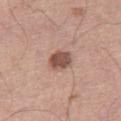Impression: The lesion was photographed on a routine skin check and not biopsied; there is no pathology result. Acquisition and patient details: From the right thigh. The patient is a male aged around 70. About 2.5 mm across. A 15 mm close-up tile from a total-body photography series done for melanoma screening.